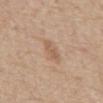Clinical impression: The lesion was tiled from a total-body skin photograph and was not biopsied. Context: A lesion tile, about 15 mm wide, cut from a 3D total-body photograph. This is a white-light tile. A male subject, in their 70s. The lesion is on the abdomen. Measured at roughly 3 mm in maximum diameter.